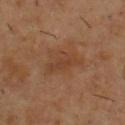Imaged during a routine full-body skin examination; the lesion was not biopsied and no histopathology is available.
A 15 mm close-up tile from a total-body photography series done for melanoma screening.
On the upper back.
The patient is a male aged approximately 55.